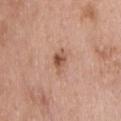Impression: This lesion was catalogued during total-body skin photography and was not selected for biopsy. Acquisition and patient details: A 15 mm close-up tile from a total-body photography series done for melanoma screening. Located on the upper back. The lesion-visualizer software estimated a footprint of about 4.5 mm², an outline eccentricity of about 0.75 (0 = round, 1 = elongated), and two-axis asymmetry of about 0.25. It also reported an automated nevus-likeness rating near 75 out of 100 and a detector confidence of about 100 out of 100 that the crop contains a lesion. About 3 mm across. The patient is a male approximately 40 years of age.